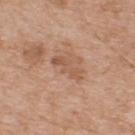{
  "biopsy_status": "not biopsied; imaged during a skin examination",
  "lesion_size": {
    "long_diameter_mm_approx": 3.5
  },
  "image": {
    "source": "total-body photography crop",
    "field_of_view_mm": 15
  },
  "lighting": "white-light",
  "site": "upper back",
  "patient": {
    "sex": "male",
    "age_approx": 55
  },
  "automated_metrics": {
    "area_mm2_approx": 5.0,
    "eccentricity": 0.9,
    "shape_asymmetry": 0.4,
    "cielab_L": 55,
    "cielab_a": 21,
    "cielab_b": 31,
    "vs_skin_darker_L": 8.0,
    "vs_skin_contrast_norm": 5.5,
    "nevus_likeness_0_100": 0,
    "lesion_detection_confidence_0_100": 100
  }
}